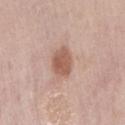This lesion was catalogued during total-body skin photography and was not selected for biopsy.
A male patient approximately 75 years of age.
A 15 mm close-up extracted from a 3D total-body photography capture.
From the lower back.